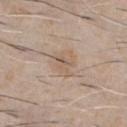Findings:
* workup · imaged on a skin check; not biopsied
* image source · 15 mm crop, total-body photography
* anatomic site · the chest
* subject · male, aged approximately 60
* illumination · white-light illumination